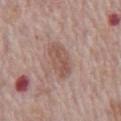The lesion was tiled from a total-body skin photograph and was not biopsied. An algorithmic analysis of the crop reported an area of roughly 9 mm², an outline eccentricity of about 0.9 (0 = round, 1 = elongated), and a shape-asymmetry score of about 0.25 (0 = symmetric). The analysis additionally found an automated nevus-likeness rating near 0 out of 100 and lesion-presence confidence of about 95/100. A region of skin cropped from a whole-body photographic capture, roughly 15 mm wide. Approximately 5 mm at its widest. The subject is a male about 70 years old. The lesion is on the abdomen.